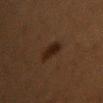notes: catalogued during a skin exam; not biopsied
location: the chest
lesion diameter: ≈3.5 mm
patient: female, about 80 years old
automated lesion analysis: a shape eccentricity near 0.9 and a symmetry-axis asymmetry near 0.15; an average lesion color of about L≈15 a*≈13 b*≈18 (CIELAB), about 6 CIELAB-L* units darker than the surrounding skin, and a normalized lesion–skin contrast near 9; a detector confidence of about 100 out of 100 that the crop contains a lesion
acquisition: total-body-photography crop, ~15 mm field of view